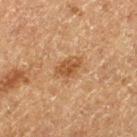A male patient aged 73 to 77. Longest diameter approximately 3.5 mm. Captured under cross-polarized illumination. The lesion is located on the left thigh. The total-body-photography lesion software estimated an area of roughly 6 mm², an outline eccentricity of about 0.75 (0 = round, 1 = elongated), and a symmetry-axis asymmetry near 0.3. And it measured a border-irregularity rating of about 3/10 and peripheral color asymmetry of about 1. Cropped from a total-body skin-imaging series; the visible field is about 15 mm.